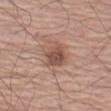<lesion>
  <biopsy_status>not biopsied; imaged during a skin examination</biopsy_status>
  <patient>
    <sex>male</sex>
    <age_approx>70</age_approx>
  </patient>
  <site>left upper arm</site>
  <lighting>white-light</lighting>
  <automated_metrics>
    <area_mm2_approx>8.0</area_mm2_approx>
    <eccentricity>0.55</eccentricity>
    <shape_asymmetry>0.2</shape_asymmetry>
    <cielab_L>51</cielab_L>
    <cielab_a>21</cielab_a>
    <cielab_b>27</cielab_b>
    <vs_skin_darker_L>11.0</vs_skin_darker_L>
    <nevus_likeness_0_100>65</nevus_likeness_0_100>
    <lesion_detection_confidence_0_100>100</lesion_detection_confidence_0_100>
  </automated_metrics>
  <lesion_size>
    <long_diameter_mm_approx>3.5</long_diameter_mm_approx>
  </lesion_size>
  <image>
    <source>total-body photography crop</source>
    <field_of_view_mm>15</field_of_view_mm>
  </image>
</lesion>